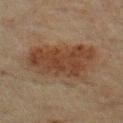<lesion>
  <automated_metrics>
    <nevus_likeness_0_100>90</nevus_likeness_0_100>
  </automated_metrics>
  <lighting>cross-polarized</lighting>
  <site>upper back</site>
  <patient>
    <sex>male</sex>
    <age_approx>65</age_approx>
  </patient>
  <image>
    <source>total-body photography crop</source>
    <field_of_view_mm>15</field_of_view_mm>
  </image>
</lesion>